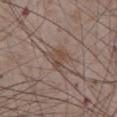<lesion>
<biopsy_status>not biopsied; imaged during a skin examination</biopsy_status>
<image>
  <source>total-body photography crop</source>
  <field_of_view_mm>15</field_of_view_mm>
</image>
<patient>
  <sex>male</sex>
  <age_approx>75</age_approx>
</patient>
<site>abdomen</site>
</lesion>